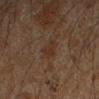Imaged during a routine full-body skin examination; the lesion was not biopsied and no histopathology is available. Cropped from a whole-body photographic skin survey; the tile spans about 15 mm. On the left forearm. A male patient aged around 45. Imaged with cross-polarized lighting. The total-body-photography lesion software estimated a footprint of about 4.5 mm², a shape eccentricity near 0.4, and two-axis asymmetry of about 0.3. The analysis additionally found a lesion color around L≈24 a*≈13 b*≈21 in CIELAB, a lesion–skin lightness drop of about 4, and a normalized border contrast of about 5.5. The software also gave internal color variation of about 2 on a 0–10 scale and radial color variation of about 1. And it measured a nevus-likeness score of about 0/100 and lesion-presence confidence of about 100/100. Measured at roughly 2.5 mm in maximum diameter.